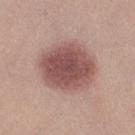workup — total-body-photography surveillance lesion; no biopsy
subject — female, in their 20s
location — the right thigh
illumination — white-light illumination
imaging modality — ~15 mm tile from a whole-body skin photo